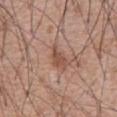The lesion was photographed on a routine skin check and not biopsied; there is no pathology result.
A male patient, approximately 60 years of age.
This is a white-light tile.
A 15 mm close-up tile from a total-body photography series done for melanoma screening.
From the abdomen.
Longest diameter approximately 3 mm.